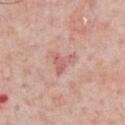Captured during whole-body skin photography for melanoma surveillance; the lesion was not biopsied. The recorded lesion diameter is about 3 mm. Located on the chest. A male patient, aged 58 to 62. A roughly 15 mm field-of-view crop from a total-body skin photograph. The tile uses white-light illumination.